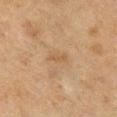Image and clinical context:
The tile uses cross-polarized illumination. An algorithmic analysis of the crop reported a lesion area of about 2.5 mm² and a shape-asymmetry score of about 0.3 (0 = symmetric). A 15 mm close-up extracted from a 3D total-body photography capture. The subject is a female aged approximately 40. The lesion is located on the front of the torso.